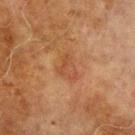Impression:
Imaged during a routine full-body skin examination; the lesion was not biopsied and no histopathology is available.
Background:
A close-up tile cropped from a whole-body skin photograph, about 15 mm across. A male subject, in their 70s. From the left upper arm. Measured at roughly 3.5 mm in maximum diameter.